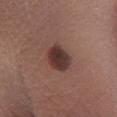follow-up = no biopsy performed (imaged during a skin exam)
image source = ~15 mm crop, total-body skin-cancer survey
patient = female, roughly 25 years of age
site = the left lower leg